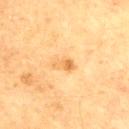{
  "lesion_size": {
    "long_diameter_mm_approx": 3.0
  },
  "image": {
    "source": "total-body photography crop",
    "field_of_view_mm": 15
  },
  "site": "arm",
  "patient": {
    "sex": "male",
    "age_approx": 65
  }
}